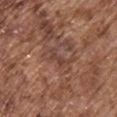follow-up: no biopsy performed (imaged during a skin exam)
patient: male, approximately 75 years of age
image: ~15 mm crop, total-body skin-cancer survey
location: the front of the torso
lesion diameter: ~3 mm (longest diameter)